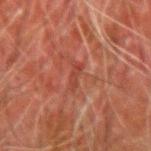biopsy status: imaged on a skin check; not biopsied | subject: male, aged around 80 | location: the right forearm | lesion diameter: ≈3 mm | image: ~15 mm tile from a whole-body skin photo | TBP lesion metrics: a lesion color around L≈34 a*≈24 b*≈26 in CIELAB and a lesion-to-skin contrast of about 5 (normalized; higher = more distinct); a border-irregularity rating of about 3.5/10, a color-variation rating of about 0/10, and radial color variation of about 0; an automated nevus-likeness rating near 0 out of 100 and a detector confidence of about 90 out of 100 that the crop contains a lesion.